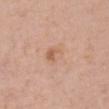<tbp_lesion>
  <automated_metrics>
    <area_mm2_approx>6.5</area_mm2_approx>
    <eccentricity>0.65</eccentricity>
    <shape_asymmetry>0.2</shape_asymmetry>
    <cielab_L>63</cielab_L>
    <cielab_a>20</cielab_a>
    <cielab_b>32</cielab_b>
    <vs_skin_darker_L>6.0</vs_skin_darker_L>
    <vs_skin_contrast_norm>4.5</vs_skin_contrast_norm>
    <border_irregularity_0_10>2.0</border_irregularity_0_10>
    <color_variation_0_10>6.0</color_variation_0_10>
    <peripheral_color_asymmetry>2.0</peripheral_color_asymmetry>
  </automated_metrics>
  <lighting>white-light</lighting>
  <image>
    <source>total-body photography crop</source>
    <field_of_view_mm>15</field_of_view_mm>
  </image>
  <site>chest</site>
  <lesion_size>
    <long_diameter_mm_approx>3.5</long_diameter_mm_approx>
  </lesion_size>
  <patient>
    <sex>male</sex>
    <age_approx>60</age_approx>
  </patient>
</tbp_lesion>